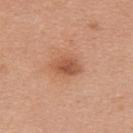Assessment:
No biopsy was performed on this lesion — it was imaged during a full skin examination and was not determined to be concerning.